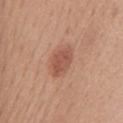Case summary:
- biopsy status — no biopsy performed (imaged during a skin exam)
- subject — female, approximately 65 years of age
- location — the mid back
- acquisition — ~15 mm crop, total-body skin-cancer survey